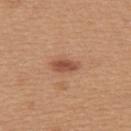Q: Is there a histopathology result?
A: total-body-photography surveillance lesion; no biopsy
Q: What is the imaging modality?
A: 15 mm crop, total-body photography
Q: What lighting was used for the tile?
A: white-light
Q: What are the patient's age and sex?
A: female, aged around 30
Q: Lesion location?
A: the upper back
Q: Automated lesion metrics?
A: an area of roughly 5 mm² and a symmetry-axis asymmetry near 0.2; a mean CIELAB color near L≈52 a*≈24 b*≈32, about 11 CIELAB-L* units darker than the surrounding skin, and a lesion-to-skin contrast of about 8 (normalized; higher = more distinct); a border-irregularity index near 2/10, a color-variation rating of about 3/10, and a peripheral color-asymmetry measure near 1
Q: Lesion size?
A: about 3.5 mm